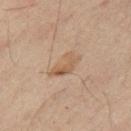No biopsy was performed on this lesion — it was imaged during a full skin examination and was not determined to be concerning. A 15 mm close-up extracted from a 3D total-body photography capture. From the arm. A male subject, roughly 50 years of age.